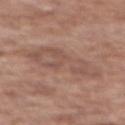lighting: white-light
image-analysis metrics: a mean CIELAB color near L≈52 a*≈19 b*≈25; a border-irregularity rating of about 5.5/10, internal color variation of about 4 on a 0–10 scale, and radial color variation of about 1; a nevus-likeness score of about 0/100 and a detector confidence of about 100 out of 100 that the crop contains a lesion
image: 15 mm crop, total-body photography
site: the back
lesion diameter: about 7 mm
subject: female, about 75 years old